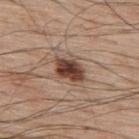The lesion was tiled from a total-body skin photograph and was not biopsied.
Cropped from a whole-body photographic skin survey; the tile spans about 15 mm.
The total-body-photography lesion software estimated a lesion area of about 9 mm², an eccentricity of roughly 0.7, and two-axis asymmetry of about 0.25. The analysis additionally found an average lesion color of about L≈44 a*≈18 b*≈25 (CIELAB), about 16 CIELAB-L* units darker than the surrounding skin, and a lesion-to-skin contrast of about 11.5 (normalized; higher = more distinct). It also reported border irregularity of about 2.5 on a 0–10 scale and a peripheral color-asymmetry measure near 1.5.
Measured at roughly 4.5 mm in maximum diameter.
The subject is a male aged approximately 70.
The lesion is located on the upper back.